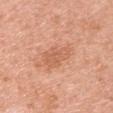follow-up: imaged on a skin check; not biopsied
subject: female, aged around 40
site: the right upper arm
image source: 15 mm crop, total-body photography
lesion diameter: ≈4 mm